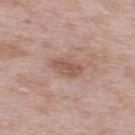Part of a total-body skin-imaging series; this lesion was reviewed on a skin check and was not flagged for biopsy.
Automated image analysis of the tile measured an area of roughly 5.5 mm², an eccentricity of roughly 0.85, and two-axis asymmetry of about 0.25. And it measured an average lesion color of about L≈54 a*≈20 b*≈27 (CIELAB), roughly 9 lightness units darker than nearby skin, and a lesion-to-skin contrast of about 6.5 (normalized; higher = more distinct).
The lesion is located on the upper back.
Imaged with white-light lighting.
A 15 mm close-up extracted from a 3D total-body photography capture.
A male patient, aged approximately 55.
The lesion's longest dimension is about 3.5 mm.